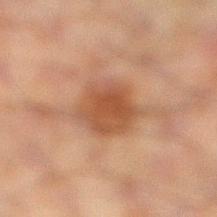No biopsy was performed on this lesion — it was imaged during a full skin examination and was not determined to be concerning. Cropped from a total-body skin-imaging series; the visible field is about 15 mm. A male subject, about 60 years old. The lesion is on the left leg. About 5 mm across.